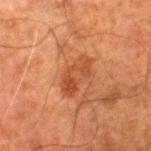notes: catalogued during a skin exam; not biopsied
diameter: ≈4.5 mm
acquisition: total-body-photography crop, ~15 mm field of view
patient: male, aged around 80
site: the right thigh
illumination: cross-polarized illumination